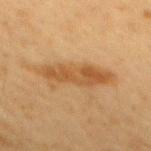| feature | finding |
|---|---|
| biopsy status | catalogued during a skin exam; not biopsied |
| site | the mid back |
| acquisition | ~15 mm crop, total-body skin-cancer survey |
| lighting | cross-polarized illumination |
| patient | male, roughly 60 years of age |